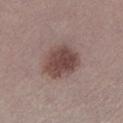Recorded during total-body skin imaging; not selected for excision or biopsy. From the leg. This image is a 15 mm lesion crop taken from a total-body photograph. The subject is a female aged 38–42.